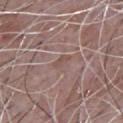Q: Is there a histopathology result?
A: catalogued during a skin exam; not biopsied
Q: Illumination type?
A: white-light
Q: Lesion location?
A: the chest
Q: Patient demographics?
A: male, aged 63 to 67
Q: What is the lesion's diameter?
A: ~2.5 mm (longest diameter)
Q: What is the imaging modality?
A: total-body-photography crop, ~15 mm field of view